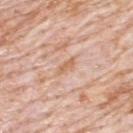Clinical impression:
The lesion was photographed on a routine skin check and not biopsied; there is no pathology result.
Context:
A close-up tile cropped from a whole-body skin photograph, about 15 mm across. On the upper back. A male patient, about 80 years old.